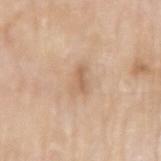Case summary:
– notes — imaged on a skin check; not biopsied
– lesion size — ≈3 mm
– image — total-body-photography crop, ~15 mm field of view
– anatomic site — the right upper arm
– subject — male, about 80 years old